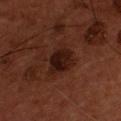lighting: cross-polarized
patient:
  sex: male
  age_approx: 55
automated_metrics:
  area_mm2_approx: 9.0
  eccentricity: 0.5
  shape_asymmetry: 0.25
  cielab_L: 16
  cielab_a: 18
  cielab_b: 19
  vs_skin_darker_L: 8.0
  border_irregularity_0_10: 2.5
  color_variation_0_10: 4.0
  peripheral_color_asymmetry: 1.5
  nevus_likeness_0_100: 75
  lesion_detection_confidence_0_100: 100
image:
  source: total-body photography crop
  field_of_view_mm: 15
lesion_size:
  long_diameter_mm_approx: 4.0
site: chest